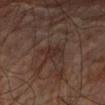Notes:
- biopsy status · total-body-photography surveillance lesion; no biopsy
- automated lesion analysis · a within-lesion color-variation index near 1.5/10; lesion-presence confidence of about 75/100
- subject · male, aged approximately 75
- illumination · cross-polarized
- image source · total-body-photography crop, ~15 mm field of view
- location · the arm